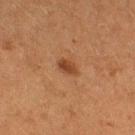| feature | finding |
|---|---|
| follow-up | total-body-photography surveillance lesion; no biopsy |
| acquisition | total-body-photography crop, ~15 mm field of view |
| subject | female, aged 48 to 52 |
| location | the right thigh |
| size | ≈2.5 mm |
| illumination | cross-polarized illumination |